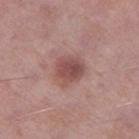| key | value |
|---|---|
| biopsy status | imaged on a skin check; not biopsied |
| automated lesion analysis | an automated nevus-likeness rating near 85 out of 100 |
| subject | male, roughly 55 years of age |
| anatomic site | the left thigh |
| imaging modality | ~15 mm crop, total-body skin-cancer survey |